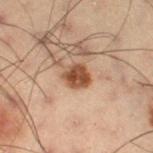{"biopsy_status": "not biopsied; imaged during a skin examination", "patient": {"sex": "male", "age_approx": 55}, "site": "leg", "image": {"source": "total-body photography crop", "field_of_view_mm": 15}}